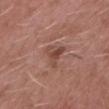Recorded during total-body skin imaging; not selected for excision or biopsy.
On the chest.
Imaged with white-light lighting.
A 15 mm close-up extracted from a 3D total-body photography capture.
A male patient, in their 50s.